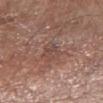Assessment:
The lesion was photographed on a routine skin check and not biopsied; there is no pathology result.
Context:
A lesion tile, about 15 mm wide, cut from a 3D total-body photograph. A male patient aged 53 to 57. On the right forearm. This is a white-light tile.